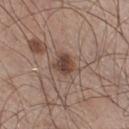A male subject, roughly 60 years of age. From the leg. Longest diameter approximately 2.5 mm. Automated image analysis of the tile measured an area of roughly 5 mm², an eccentricity of roughly 0.5, and a shape-asymmetry score of about 0.25 (0 = symmetric). It also reported a nevus-likeness score of about 85/100 and lesion-presence confidence of about 100/100. A lesion tile, about 15 mm wide, cut from a 3D total-body photograph.